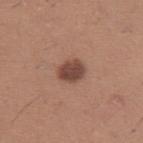Recorded during total-body skin imaging; not selected for excision or biopsy. From the left upper arm. The subject is a male in their mid- to late 30s. The lesion-visualizer software estimated a footprint of about 6.5 mm² and a shape eccentricity near 0.6. The software also gave an automated nevus-likeness rating near 80 out of 100 and lesion-presence confidence of about 100/100. A 15 mm crop from a total-body photograph taken for skin-cancer surveillance. Measured at roughly 3 mm in maximum diameter.